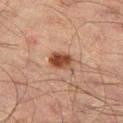The lesion was tiled from a total-body skin photograph and was not biopsied. The patient is a male aged around 50. Longest diameter approximately 3 mm. Automated tile analysis of the lesion measured an area of roughly 6 mm² and a shape eccentricity near 0.75. The software also gave an average lesion color of about L≈36 a*≈19 b*≈26 (CIELAB), a lesion–skin lightness drop of about 12, and a normalized lesion–skin contrast near 10.5. The analysis additionally found a border-irregularity rating of about 1.5/10, a within-lesion color-variation index near 4.5/10, and a peripheral color-asymmetry measure near 1.5. The tile uses cross-polarized illumination. A roughly 15 mm field-of-view crop from a total-body skin photograph. The lesion is on the leg.